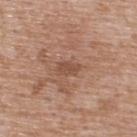<lesion>
<biopsy_status>not biopsied; imaged during a skin examination</biopsy_status>
<lighting>white-light</lighting>
<site>upper back</site>
<lesion_size>
  <long_diameter_mm_approx>2.5</long_diameter_mm_approx>
</lesion_size>
<image>
  <source>total-body photography crop</source>
  <field_of_view_mm>15</field_of_view_mm>
</image>
<patient>
  <sex>male</sex>
  <age_approx>50</age_approx>
</patient>
<automated_metrics>
  <lesion_detection_confidence_0_100>100</lesion_detection_confidence_0_100>
</automated_metrics>
</lesion>